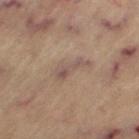Notes:
* follow-up · total-body-photography surveillance lesion; no biopsy
* TBP lesion metrics · a footprint of about 6.5 mm² and a symmetry-axis asymmetry near 0.4
* diameter · ~4.5 mm (longest diameter)
* subject · female, aged approximately 65
* site · the left thigh
* imaging modality · 15 mm crop, total-body photography
* illumination · cross-polarized